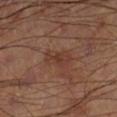Captured during whole-body skin photography for melanoma surveillance; the lesion was not biopsied. A 15 mm close-up tile from a total-body photography series done for melanoma screening. The tile uses cross-polarized illumination. On the right lower leg.